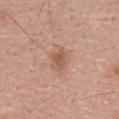| feature | finding |
|---|---|
| image source | 15 mm crop, total-body photography |
| location | the abdomen |
| subject | male, aged 53–57 |